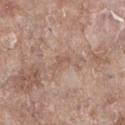Q: Is there a histopathology result?
A: total-body-photography surveillance lesion; no biopsy
Q: What are the patient's age and sex?
A: female, in their mid-70s
Q: How was the tile lit?
A: white-light
Q: How was this image acquired?
A: total-body-photography crop, ~15 mm field of view
Q: Where on the body is the lesion?
A: the left lower leg
Q: How large is the lesion?
A: about 3 mm